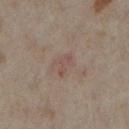A region of skin cropped from a whole-body photographic capture, roughly 15 mm wide.
A female subject, aged 33 to 37.
On the left lower leg.